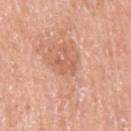  biopsy_status: not biopsied; imaged during a skin examination
  patient:
    sex: male
    age_approx: 60
  lighting: white-light
  lesion_size:
    long_diameter_mm_approx: 2.5
  site: left upper arm
  image:
    source: total-body photography crop
    field_of_view_mm: 15